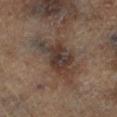The lesion is located on the leg. Measured at roughly 7.5 mm in maximum diameter. A roughly 15 mm field-of-view crop from a total-body skin photograph. A male subject, roughly 65 years of age. Captured under cross-polarized illumination.